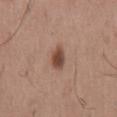<record>
<site>mid back</site>
<patient>
  <sex>male</sex>
  <age_approx>65</age_approx>
</patient>
<lesion_size>
  <long_diameter_mm_approx>3.0</long_diameter_mm_approx>
</lesion_size>
<image>
  <source>total-body photography crop</source>
  <field_of_view_mm>15</field_of_view_mm>
</image>
</record>